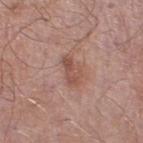Recorded during total-body skin imaging; not selected for excision or biopsy. The lesion-visualizer software estimated an area of roughly 5 mm², a shape eccentricity near 0.85, and a symmetry-axis asymmetry near 0.25. And it measured a border-irregularity index near 3/10, a color-variation rating of about 2.5/10, and a peripheral color-asymmetry measure near 0.5. The subject is a male roughly 55 years of age. A close-up tile cropped from a whole-body skin photograph, about 15 mm across. On the left lower leg.